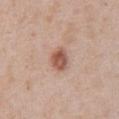No biopsy was performed on this lesion — it was imaged during a full skin examination and was not determined to be concerning.
Imaged with white-light lighting.
A male subject in their mid-60s.
This image is a 15 mm lesion crop taken from a total-body photograph.
The lesion is on the abdomen.